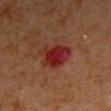Q: Was this lesion biopsied?
A: imaged on a skin check; not biopsied
Q: What is the anatomic site?
A: the right upper arm
Q: Illumination type?
A: cross-polarized
Q: How was this image acquired?
A: total-body-photography crop, ~15 mm field of view
Q: Lesion size?
A: ~4 mm (longest diameter)
Q: Patient demographics?
A: male, aged 63 to 67